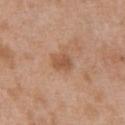This lesion was catalogued during total-body skin photography and was not selected for biopsy.
The lesion is on the chest.
The subject is a female about 40 years old.
Automated tile analysis of the lesion measured a border-irregularity index near 2.5/10 and a peripheral color-asymmetry measure near 0.5. The software also gave an automated nevus-likeness rating near 5 out of 100 and lesion-presence confidence of about 100/100.
About 2.5 mm across.
A 15 mm crop from a total-body photograph taken for skin-cancer surveillance.